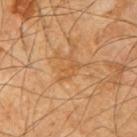Q: What is the anatomic site?
A: the arm
Q: What are the patient's age and sex?
A: male, about 60 years old
Q: What kind of image is this?
A: ~15 mm crop, total-body skin-cancer survey
Q: What is the lesion's diameter?
A: ~3.5 mm (longest diameter)
Q: What lighting was used for the tile?
A: cross-polarized